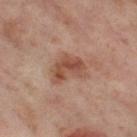{
  "biopsy_status": "not biopsied; imaged during a skin examination",
  "site": "leg",
  "lesion_size": {
    "long_diameter_mm_approx": 4.0
  },
  "patient": {
    "sex": "female",
    "age_approx": 55
  },
  "image": {
    "source": "total-body photography crop",
    "field_of_view_mm": 15
  },
  "automated_metrics": {
    "cielab_L": 50,
    "cielab_a": 22,
    "cielab_b": 29,
    "vs_skin_darker_L": 10.0,
    "vs_skin_contrast_norm": 7.5,
    "border_irregularity_0_10": 4.5,
    "peripheral_color_asymmetry": 1.5,
    "nevus_likeness_0_100": 55,
    "lesion_detection_confidence_0_100": 100
  }
}